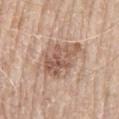Part of a total-body skin-imaging series; this lesion was reviewed on a skin check and was not flagged for biopsy.
A male subject roughly 80 years of age.
The lesion-visualizer software estimated a symmetry-axis asymmetry near 0.25. The analysis additionally found a nevus-likeness score of about 20/100.
Approximately 6.5 mm at its widest.
A 15 mm crop from a total-body photograph taken for skin-cancer surveillance.
The tile uses white-light illumination.
From the mid back.